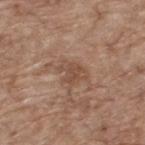This lesion was catalogued during total-body skin photography and was not selected for biopsy. The lesion's longest dimension is about 3.5 mm. A roughly 15 mm field-of-view crop from a total-body skin photograph. The lesion is located on the upper back. Automated image analysis of the tile measured an average lesion color of about L≈48 a*≈19 b*≈28 (CIELAB) and about 7 CIELAB-L* units darker than the surrounding skin. The analysis additionally found a border-irregularity rating of about 4.5/10, a within-lesion color-variation index near 1.5/10, and radial color variation of about 0.5. A male patient, about 70 years old.